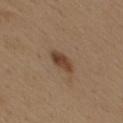| key | value |
|---|---|
| notes | catalogued during a skin exam; not biopsied |
| body site | the mid back |
| patient | female, aged approximately 40 |
| size | ≈3.5 mm |
| image source | 15 mm crop, total-body photography |
| lighting | white-light |